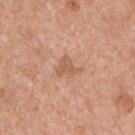Impression:
The lesion was photographed on a routine skin check and not biopsied; there is no pathology result.
Clinical summary:
Imaged with white-light lighting. Cropped from a whole-body photographic skin survey; the tile spans about 15 mm. The recorded lesion diameter is about 3 mm. Automated image analysis of the tile measured a mean CIELAB color near L≈58 a*≈22 b*≈33 and about 8 CIELAB-L* units darker than the surrounding skin. The analysis additionally found a border-irregularity rating of about 5.5/10 and radial color variation of about 0.5. The lesion is on the front of the torso. The subject is a male about 55 years old.